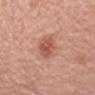Clinical impression: The lesion was tiled from a total-body skin photograph and was not biopsied. Background: The lesion is on the left upper arm. A region of skin cropped from a whole-body photographic capture, roughly 15 mm wide. A female patient, aged around 65.